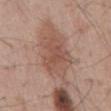Findings:
* notes — total-body-photography surveillance lesion; no biopsy
* anatomic site — the abdomen
* image — ~15 mm crop, total-body skin-cancer survey
* image-analysis metrics — roughly 8 lightness units darker than nearby skin and a normalized lesion–skin contrast near 6; border irregularity of about 3.5 on a 0–10 scale and internal color variation of about 3.5 on a 0–10 scale
* tile lighting — white-light illumination
* size — ≈5.5 mm
* subject — male, about 65 years old